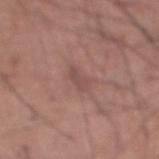follow-up: total-body-photography surveillance lesion; no biopsy
subject: male, aged around 55
lesion size: about 3 mm
image source: ~15 mm tile from a whole-body skin photo
site: the abdomen